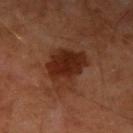No biopsy was performed on this lesion — it was imaged during a full skin examination and was not determined to be concerning. The patient is a male aged approximately 65. A lesion tile, about 15 mm wide, cut from a 3D total-body photograph. From the right upper arm. Captured under cross-polarized illumination. The lesion-visualizer software estimated an area of roughly 16 mm² and two-axis asymmetry of about 0.3. It also reported a lesion color around L≈27 a*≈23 b*≈28 in CIELAB and roughly 10 lightness units darker than nearby skin. The analysis additionally found a border-irregularity rating of about 3.5/10, a within-lesion color-variation index near 4.5/10, and radial color variation of about 1.5. Approximately 5 mm at its widest.